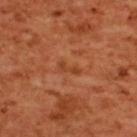{
  "biopsy_status": "not biopsied; imaged during a skin examination",
  "site": "upper back",
  "patient": {
    "sex": "female",
    "age_approx": 55
  },
  "lighting": "cross-polarized",
  "automated_metrics": {
    "cielab_L": 45,
    "cielab_a": 30,
    "cielab_b": 39,
    "vs_skin_darker_L": 7.0,
    "vs_skin_contrast_norm": 6.0,
    "border_irregularity_0_10": 5.0,
    "color_variation_0_10": 0.0,
    "peripheral_color_asymmetry": 0.0,
    "lesion_detection_confidence_0_100": 100
  },
  "image": {
    "source": "total-body photography crop",
    "field_of_view_mm": 15
  }
}